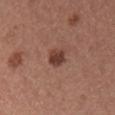biopsy status: catalogued during a skin exam; not biopsied
acquisition: ~15 mm crop, total-body skin-cancer survey
lesion size: ≈2.5 mm
site: the chest
illumination: white-light
patient: male, about 40 years old